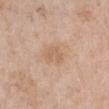Clinical impression: Part of a total-body skin-imaging series; this lesion was reviewed on a skin check and was not flagged for biopsy. Background: Cropped from a whole-body photographic skin survey; the tile spans about 15 mm. This is a white-light tile. The lesion is located on the right lower leg. The patient is a female aged 68–72.